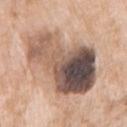| key | value |
|---|---|
| biopsy status | imaged on a skin check; not biopsied |
| diameter | about 11.5 mm |
| body site | the right upper arm |
| illumination | white-light illumination |
| imaging modality | total-body-photography crop, ~15 mm field of view |
| subject | female, roughly 75 years of age |